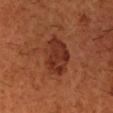workup — total-body-photography surveillance lesion; no biopsy
lesion diameter — ~5.5 mm (longest diameter)
imaging modality — 15 mm crop, total-body photography
TBP lesion metrics — a footprint of about 13 mm² and an outline eccentricity of about 0.8 (0 = round, 1 = elongated); a border-irregularity rating of about 2.5/10, internal color variation of about 3.5 on a 0–10 scale, and radial color variation of about 1; an automated nevus-likeness rating near 65 out of 100 and a detector confidence of about 100 out of 100 that the crop contains a lesion
patient — male, aged around 60
site — the head or neck